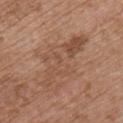biopsy status: no biopsy performed (imaged during a skin exam) | location: the chest | imaging modality: ~15 mm tile from a whole-body skin photo | patient: male, in their mid-60s.